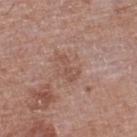workup: total-body-photography surveillance lesion; no biopsy | imaging modality: ~15 mm crop, total-body skin-cancer survey | tile lighting: white-light illumination | site: the right lower leg | subject: female, about 70 years old.